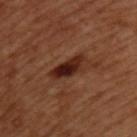Assessment:
The lesion was tiled from a total-body skin photograph and was not biopsied.
Clinical summary:
The subject is a male approximately 50 years of age. A 15 mm crop from a total-body photograph taken for skin-cancer surveillance. The lesion is on the upper back. This is a cross-polarized tile.